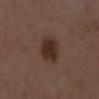Clinical impression: Recorded during total-body skin imaging; not selected for excision or biopsy.